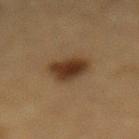Q: Is there a histopathology result?
A: catalogued during a skin exam; not biopsied
Q: What lighting was used for the tile?
A: cross-polarized illumination
Q: Automated lesion metrics?
A: a footprint of about 10 mm², an eccentricity of roughly 0.45, and two-axis asymmetry of about 0.2; about 11 CIELAB-L* units darker than the surrounding skin; border irregularity of about 2 on a 0–10 scale, internal color variation of about 4 on a 0–10 scale, and a peripheral color-asymmetry measure near 1
Q: Who is the patient?
A: male, approximately 85 years of age or older
Q: What kind of image is this?
A: total-body-photography crop, ~15 mm field of view
Q: What is the anatomic site?
A: the mid back
Q: Lesion size?
A: ~3.5 mm (longest diameter)